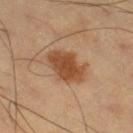Imaged during a routine full-body skin examination; the lesion was not biopsied and no histopathology is available. A male patient about 60 years old. This is a cross-polarized tile. Approximately 4.5 mm at its widest. On the leg. The lesion-visualizer software estimated an area of roughly 12 mm², an outline eccentricity of about 0.7 (0 = round, 1 = elongated), and a shape-asymmetry score of about 0.2 (0 = symmetric). It also reported a classifier nevus-likeness of about 90/100. Cropped from a whole-body photographic skin survey; the tile spans about 15 mm.